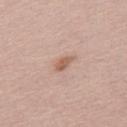Case summary:
* biopsy status — total-body-photography surveillance lesion; no biopsy
* lesion diameter — ≈2.5 mm
* image — ~15 mm crop, total-body skin-cancer survey
* patient — male, in their 30s
* image-analysis metrics — a mean CIELAB color near L≈59 a*≈20 b*≈28 and a lesion–skin lightness drop of about 10; border irregularity of about 2.5 on a 0–10 scale, a within-lesion color-variation index near 1.5/10, and a peripheral color-asymmetry measure near 0.5; a classifier nevus-likeness of about 45/100 and a lesion-detection confidence of about 100/100
* lighting — white-light